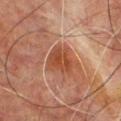Notes:
* notes — imaged on a skin check; not biopsied
* lighting — cross-polarized illumination
* subject — male, aged 63–67
* size — ~3.5 mm (longest diameter)
* body site — the chest
* acquisition — total-body-photography crop, ~15 mm field of view